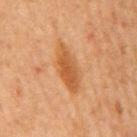<case>
<biopsy_status>not biopsied; imaged during a skin examination</biopsy_status>
<lighting>cross-polarized</lighting>
<site>back</site>
<image>
  <source>total-body photography crop</source>
  <field_of_view_mm>15</field_of_view_mm>
</image>
<patient>
  <sex>male</sex>
  <age_approx>65</age_approx>
</patient>
</case>